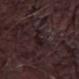* location: the left thigh
* subject: male, about 30 years old
* acquisition: 15 mm crop, total-body photography
* illumination: white-light illumination
* lesion size: about 3.5 mm